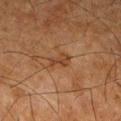{"biopsy_status": "not biopsied; imaged during a skin examination", "lesion_size": {"long_diameter_mm_approx": 3.0}, "image": {"source": "total-body photography crop", "field_of_view_mm": 15}, "patient": {"sex": "male", "age_approx": 80}, "lighting": "cross-polarized", "site": "right thigh"}